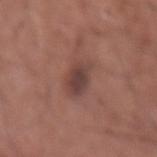| field | value |
|---|---|
| biopsy status | no biopsy performed (imaged during a skin exam) |
| subject | male, aged approximately 55 |
| lighting | white-light |
| automated lesion analysis | a mean CIELAB color near L≈41 a*≈20 b*≈22, about 9 CIELAB-L* units darker than the surrounding skin, and a normalized border contrast of about 8 |
| anatomic site | the right forearm |
| imaging modality | ~15 mm crop, total-body skin-cancer survey |